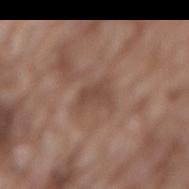| field | value |
|---|---|
| biopsy status | catalogued during a skin exam; not biopsied |
| tile lighting | white-light |
| lesion diameter | ~3 mm (longest diameter) |
| image | ~15 mm tile from a whole-body skin photo |
| automated metrics | an average lesion color of about L≈45 a*≈19 b*≈25 (CIELAB) and a normalized border contrast of about 6; border irregularity of about 4.5 on a 0–10 scale and a within-lesion color-variation index near 1/10; a nevus-likeness score of about 0/100 and a detector confidence of about 95 out of 100 that the crop contains a lesion |
| subject | male, approximately 75 years of age |
| site | the back |